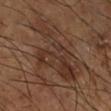Imaged during a routine full-body skin examination; the lesion was not biopsied and no histopathology is available.
On the right lower leg.
The recorded lesion diameter is about 9 mm.
Automated tile analysis of the lesion measured a lesion color around L≈33 a*≈18 b*≈25 in CIELAB and roughly 7 lightness units darker than nearby skin. The software also gave border irregularity of about 9.5 on a 0–10 scale, a within-lesion color-variation index near 4.5/10, and a peripheral color-asymmetry measure near 1.5. It also reported a nevus-likeness score of about 0/100 and a lesion-detection confidence of about 80/100.
The subject is a male aged approximately 65.
A roughly 15 mm field-of-view crop from a total-body skin photograph.
Captured under cross-polarized illumination.